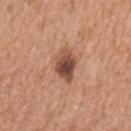Captured during whole-body skin photography for melanoma surveillance; the lesion was not biopsied.
Longest diameter approximately 4 mm.
Located on the arm.
A female subject, in their mid- to late 70s.
The tile uses white-light illumination.
A 15 mm crop from a total-body photograph taken for skin-cancer surveillance.